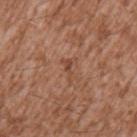This lesion was catalogued during total-body skin photography and was not selected for biopsy.
From the left upper arm.
Approximately 3 mm at its widest.
A male subject approximately 45 years of age.
Automated tile analysis of the lesion measured an area of roughly 2.5 mm², a shape eccentricity near 0.9, and a shape-asymmetry score of about 0.6 (0 = symmetric). And it measured an average lesion color of about L≈46 a*≈22 b*≈30 (CIELAB) and a normalized border contrast of about 5.5.
A 15 mm close-up extracted from a 3D total-body photography capture.